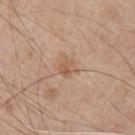<case>
<patient>
  <sex>male</sex>
  <age_approx>65</age_approx>
</patient>
<site>chest</site>
<lighting>white-light</lighting>
<lesion_size>
  <long_diameter_mm_approx>2.5</long_diameter_mm_approx>
</lesion_size>
<image>
  <source>total-body photography crop</source>
  <field_of_view_mm>15</field_of_view_mm>
</image>
</case>